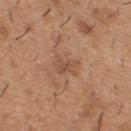The lesion is located on the upper back. Captured under white-light illumination. The subject is a male aged 38 to 42. A 15 mm crop from a total-body photograph taken for skin-cancer surveillance.